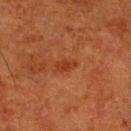Acquisition and patient details: A male patient aged 78 to 82. A 15 mm crop from a total-body photograph taken for skin-cancer surveillance. Located on the leg.